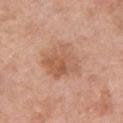This lesion was catalogued during total-body skin photography and was not selected for biopsy.
Cropped from a total-body skin-imaging series; the visible field is about 15 mm.
The recorded lesion diameter is about 5 mm.
The lesion is on the left upper arm.
Captured under white-light illumination.
The patient is a female aged approximately 60.